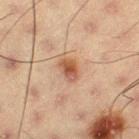Clinical impression: Captured during whole-body skin photography for melanoma surveillance; the lesion was not biopsied. Context: A male patient about 55 years old. The lesion's longest dimension is about 3 mm. Located on the leg. Cropped from a whole-body photographic skin survey; the tile spans about 15 mm. Captured under cross-polarized illumination.